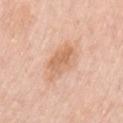Assessment: The lesion was tiled from a total-body skin photograph and was not biopsied. Image and clinical context: The recorded lesion diameter is about 4 mm. Located on the arm. A roughly 15 mm field-of-view crop from a total-body skin photograph. The subject is a female approximately 65 years of age.